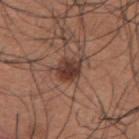Q: Is there a histopathology result?
A: catalogued during a skin exam; not biopsied
Q: How large is the lesion?
A: ≈3.5 mm
Q: Lesion location?
A: the upper back
Q: What kind of image is this?
A: ~15 mm crop, total-body skin-cancer survey
Q: How was the tile lit?
A: white-light illumination
Q: What are the patient's age and sex?
A: male, aged 33–37